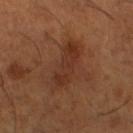Q: Was this lesion biopsied?
A: catalogued during a skin exam; not biopsied
Q: What is the anatomic site?
A: the left lower leg
Q: What lighting was used for the tile?
A: cross-polarized
Q: Lesion size?
A: ~6 mm (longest diameter)
Q: What kind of image is this?
A: ~15 mm crop, total-body skin-cancer survey
Q: What are the patient's age and sex?
A: male, in their mid- to late 60s
Q: Automated lesion metrics?
A: a lesion area of about 12 mm², a shape eccentricity near 0.95, and a symmetry-axis asymmetry near 0.25; a normalized border contrast of about 6.5; border irregularity of about 4 on a 0–10 scale and radial color variation of about 1.5; an automated nevus-likeness rating near 5 out of 100 and a lesion-detection confidence of about 100/100